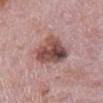Impression: Recorded during total-body skin imaging; not selected for excision or biopsy. Context: Located on the mid back. This is a white-light tile. The lesion's longest dimension is about 5.5 mm. A male patient roughly 75 years of age. A lesion tile, about 15 mm wide, cut from a 3D total-body photograph. Automated tile analysis of the lesion measured a footprint of about 16 mm². The analysis additionally found a lesion-to-skin contrast of about 10 (normalized; higher = more distinct).